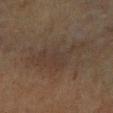Imaged during a routine full-body skin examination; the lesion was not biopsied and no histopathology is available. The total-body-photography lesion software estimated a footprint of about 21 mm² and an eccentricity of roughly 0.85. It also reported a classifier nevus-likeness of about 0/100 and lesion-presence confidence of about 65/100. A female subject, aged approximately 65. A 15 mm close-up extracted from a 3D total-body photography capture. The lesion's longest dimension is about 8 mm. Located on the left forearm.